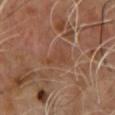Imaged during a routine full-body skin examination; the lesion was not biopsied and no histopathology is available.
A male subject, aged approximately 65.
Measured at roughly 3 mm in maximum diameter.
Automated image analysis of the tile measured a border-irregularity index near 4.5/10, internal color variation of about 1.5 on a 0–10 scale, and peripheral color asymmetry of about 0.5.
A roughly 15 mm field-of-view crop from a total-body skin photograph.
The lesion is on the chest.